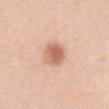biopsy status: no biopsy performed (imaged during a skin exam); patient: female, roughly 40 years of age; location: the abdomen; image: total-body-photography crop, ~15 mm field of view; tile lighting: white-light; lesion size: ≈3 mm.